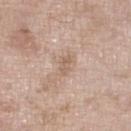Q: Was this lesion biopsied?
A: no biopsy performed (imaged during a skin exam)
Q: Patient demographics?
A: female, aged 73–77
Q: How was this image acquired?
A: total-body-photography crop, ~15 mm field of view
Q: How large is the lesion?
A: ≈2.5 mm
Q: Illumination type?
A: white-light
Q: What is the anatomic site?
A: the right lower leg